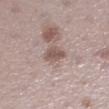Recorded during total-body skin imaging; not selected for excision or biopsy. Automated tile analysis of the lesion measured a border-irregularity rating of about 3/10 and a within-lesion color-variation index near 2.5/10. A female subject, aged 53 to 57. This is a white-light tile. Cropped from a whole-body photographic skin survey; the tile spans about 15 mm. About 3 mm across. The lesion is located on the left lower leg.